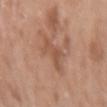Impression:
Captured during whole-body skin photography for melanoma surveillance; the lesion was not biopsied.
Clinical summary:
The lesion is located on the right upper arm. The lesion's longest dimension is about 4 mm. This image is a 15 mm lesion crop taken from a total-body photograph. The patient is a female in their mid-80s or older.